The lesion is located on the head or neck. A male patient, aged 58 to 62. A 15 mm crop from a total-body photograph taken for skin-cancer surveillance — 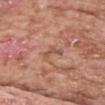Histopathological examination showed a nodular basal cell carcinoma (malignant).